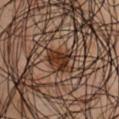Assessment:
Captured during whole-body skin photography for melanoma surveillance; the lesion was not biopsied.
Context:
The recorded lesion diameter is about 2.5 mm. Cropped from a total-body skin-imaging series; the visible field is about 15 mm. A male patient, about 45 years old. Located on the chest. Captured under cross-polarized illumination. The total-body-photography lesion software estimated a footprint of about 5 mm², a shape eccentricity near 0.5, and a shape-asymmetry score of about 0.35 (0 = symmetric). And it measured a border-irregularity rating of about 3.5/10 and a peripheral color-asymmetry measure near 1. The software also gave a nevus-likeness score of about 90/100 and lesion-presence confidence of about 100/100.